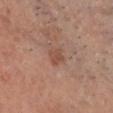Image and clinical context:
The recorded lesion diameter is about 2.5 mm. A male subject, aged approximately 45. A region of skin cropped from a whole-body photographic capture, roughly 15 mm wide. From the head or neck. This is a white-light tile.